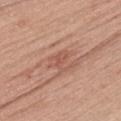Part of a total-body skin-imaging series; this lesion was reviewed on a skin check and was not flagged for biopsy.